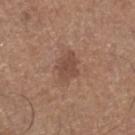<lesion>
<biopsy_status>not biopsied; imaged during a skin examination</biopsy_status>
<lighting>white-light</lighting>
<site>right lower leg</site>
<image>
  <source>total-body photography crop</source>
  <field_of_view_mm>15</field_of_view_mm>
</image>
<automated_metrics>
  <cielab_L>47</cielab_L>
  <cielab_a>19</cielab_a>
  <cielab_b>27</cielab_b>
  <border_irregularity_0_10>2.5</border_irregularity_0_10>
  <peripheral_color_asymmetry>0.5</peripheral_color_asymmetry>
  <nevus_likeness_0_100>20</nevus_likeness_0_100>
</automated_metrics>
<lesion_size>
  <long_diameter_mm_approx>3.5</long_diameter_mm_approx>
</lesion_size>
<patient>
  <sex>male</sex>
  <age_approx>75</age_approx>
</patient>
</lesion>